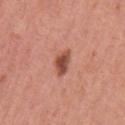Recorded during total-body skin imaging; not selected for excision or biopsy. The lesion is located on the left upper arm. This is a white-light tile. The recorded lesion diameter is about 3 mm. A close-up tile cropped from a whole-body skin photograph, about 15 mm across. The total-body-photography lesion software estimated an area of roughly 4.5 mm² and an outline eccentricity of about 0.75 (0 = round, 1 = elongated). And it measured a lesion color around L≈50 a*≈27 b*≈30 in CIELAB and a lesion–skin lightness drop of about 14. It also reported a peripheral color-asymmetry measure near 1. The analysis additionally found a nevus-likeness score of about 95/100 and a detector confidence of about 100 out of 100 that the crop contains a lesion. A female patient aged 48–52.